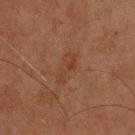Q: Was this lesion biopsied?
A: catalogued during a skin exam; not biopsied
Q: Illumination type?
A: cross-polarized
Q: Automated lesion metrics?
A: a nevus-likeness score of about 0/100
Q: Where on the body is the lesion?
A: the back
Q: How was this image acquired?
A: total-body-photography crop, ~15 mm field of view
Q: What is the lesion's diameter?
A: about 4 mm
Q: Who is the patient?
A: male, in their mid-40s